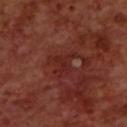workup: catalogued during a skin exam; not biopsied
image-analysis metrics: a lesion area of about 5 mm² and a shape-asymmetry score of about 0.6 (0 = symmetric)
image source: 15 mm crop, total-body photography
body site: the upper back
lesion diameter: ~3.5 mm (longest diameter)
illumination: cross-polarized illumination
subject: male, aged around 70